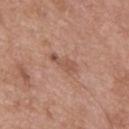notes = total-body-photography surveillance lesion; no biopsy | tile lighting = white-light | image-analysis metrics = a lesion area of about 5 mm², a shape eccentricity near 0.95, and two-axis asymmetry of about 0.35; an automated nevus-likeness rating near 0 out of 100 and a lesion-detection confidence of about 100/100 | anatomic site = the mid back | patient = male, roughly 50 years of age | imaging modality = 15 mm crop, total-body photography.